The total-body-photography lesion software estimated a lesion color around L≈31 a*≈19 b*≈19 in CIELAB, about 5 CIELAB-L* units darker than the surrounding skin, and a normalized border contrast of about 5. And it measured a within-lesion color-variation index near 2.5/10 and peripheral color asymmetry of about 1. A region of skin cropped from a whole-body photographic capture, roughly 15 mm wide. From the chest. This is a white-light tile. Longest diameter approximately 7 mm. The patient is a male about 55 years old.
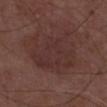On excision, pathology confirmed a lichen planus-like keratosis (benign).